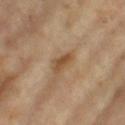The lesion was tiled from a total-body skin photograph and was not biopsied.
On the right thigh.
A roughly 15 mm field-of-view crop from a total-body skin photograph.
This is a cross-polarized tile.
The subject is a female roughly 75 years of age.
An algorithmic analysis of the crop reported a lesion area of about 4 mm² and an outline eccentricity of about 0.9 (0 = round, 1 = elongated).